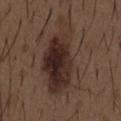follow-up — total-body-photography surveillance lesion; no biopsy | image — total-body-photography crop, ~15 mm field of view | image-analysis metrics — internal color variation of about 7.5 on a 0–10 scale and a peripheral color-asymmetry measure near 2.5; a nevus-likeness score of about 90/100 and a lesion-detection confidence of about 100/100 | size — about 11 mm | subject — male, aged 48 to 52 | illumination — white-light illumination | site — the chest.